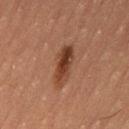body site: the right thigh | subject: male, in their mid-50s | acquisition: ~15 mm crop, total-body skin-cancer survey | diameter: about 5 mm | image-analysis metrics: an average lesion color of about L≈34 a*≈20 b*≈27 (CIELAB), roughly 11 lightness units darker than nearby skin, and a normalized border contrast of about 9.5; an automated nevus-likeness rating near 100 out of 100 and lesion-presence confidence of about 100/100 | lighting: cross-polarized.